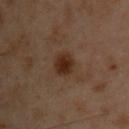| field | value |
|---|---|
| biopsy status | catalogued during a skin exam; not biopsied |
| image | ~15 mm crop, total-body skin-cancer survey |
| site | the chest |
| subject | male, aged 53–57 |
| diameter | ~3 mm (longest diameter) |